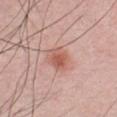follow-up: imaged on a skin check; not biopsied
image source: total-body-photography crop, ~15 mm field of view
automated metrics: a footprint of about 6.5 mm², an outline eccentricity of about 0.55 (0 = round, 1 = elongated), and a symmetry-axis asymmetry near 0.2; an average lesion color of about L≈58 a*≈25 b*≈28 (CIELAB), a lesion–skin lightness drop of about 10, and a normalized lesion–skin contrast near 7; border irregularity of about 2 on a 0–10 scale, a color-variation rating of about 3.5/10, and a peripheral color-asymmetry measure near 1
location: the chest
size: ≈3 mm
patient: male, in their 30s